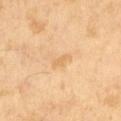Captured during whole-body skin photography for melanoma surveillance; the lesion was not biopsied.
A lesion tile, about 15 mm wide, cut from a 3D total-body photograph.
A male subject about 65 years old.
Imaged with cross-polarized lighting.
The lesion-visualizer software estimated a lesion area of about 3 mm², a shape eccentricity near 0.8, and a shape-asymmetry score of about 0.35 (0 = symmetric). And it measured a mean CIELAB color near L≈69 a*≈18 b*≈42, roughly 6 lightness units darker than nearby skin, and a normalized border contrast of about 5. The analysis additionally found a lesion-detection confidence of about 100/100.